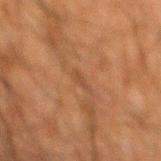  biopsy_status: not biopsied; imaged during a skin examination
  image:
    source: total-body photography crop
    field_of_view_mm: 15
  site: mid back
  lighting: cross-polarized
  patient:
    sex: male
    age_approx: 60